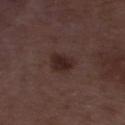notes: imaged on a skin check; not biopsied
lesion diameter: about 3 mm
automated metrics: a footprint of about 6 mm², a shape eccentricity near 0.7, and a shape-asymmetry score of about 0.3 (0 = symmetric); about 8 CIELAB-L* units darker than the surrounding skin; border irregularity of about 2.5 on a 0–10 scale and peripheral color asymmetry of about 1; a nevus-likeness score of about 85/100 and a lesion-detection confidence of about 100/100
lighting: white-light illumination
subject: male, about 50 years old
imaging modality: total-body-photography crop, ~15 mm field of view